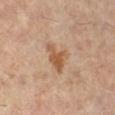Recorded during total-body skin imaging; not selected for excision or biopsy.
Captured under cross-polarized illumination.
A female subject in their 60s.
From the right lower leg.
A roughly 15 mm field-of-view crop from a total-body skin photograph.
Automated tile analysis of the lesion measured a footprint of about 7 mm², an eccentricity of roughly 0.8, and two-axis asymmetry of about 0.45. The analysis additionally found border irregularity of about 5 on a 0–10 scale, a color-variation rating of about 3.5/10, and peripheral color asymmetry of about 1.
The recorded lesion diameter is about 4.5 mm.